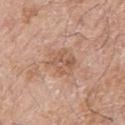Assessment:
Captured during whole-body skin photography for melanoma surveillance; the lesion was not biopsied.
Acquisition and patient details:
The total-body-photography lesion software estimated a footprint of about 8.5 mm², an outline eccentricity of about 0.55 (0 = round, 1 = elongated), and a symmetry-axis asymmetry near 0.3. The lesion is on the right thigh. This is a white-light tile. A close-up tile cropped from a whole-body skin photograph, about 15 mm across. The subject is a male aged 58 to 62.